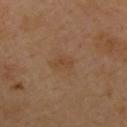The lesion was tiled from a total-body skin photograph and was not biopsied. Imaged with cross-polarized lighting. Approximately 3 mm at its widest. From the upper back. Cropped from a total-body skin-imaging series; the visible field is about 15 mm. A male subject approximately 40 years of age.